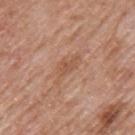Part of a total-body skin-imaging series; this lesion was reviewed on a skin check and was not flagged for biopsy.
A male patient, aged 58–62.
Located on the mid back.
A 15 mm crop from a total-body photograph taken for skin-cancer surveillance.